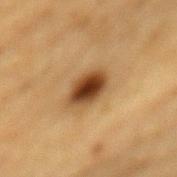<case>
<biopsy_status>not biopsied; imaged during a skin examination</biopsy_status>
<patient>
  <sex>male</sex>
  <age_approx>85+</age_approx>
</patient>
<lighting>cross-polarized</lighting>
<site>mid back</site>
<lesion_size>
  <long_diameter_mm_approx>4.0</long_diameter_mm_approx>
</lesion_size>
<image>
  <source>total-body photography crop</source>
  <field_of_view_mm>15</field_of_view_mm>
</image>
</case>